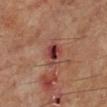The lesion was photographed on a routine skin check and not biopsied; there is no pathology result.
A male subject aged 58 to 62.
From the left lower leg.
A 15 mm close-up tile from a total-body photography series done for melanoma screening.